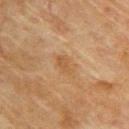The lesion was tiled from a total-body skin photograph and was not biopsied.
A female patient aged approximately 80.
Measured at roughly 2.5 mm in maximum diameter.
The lesion is on the upper back.
The tile uses cross-polarized illumination.
A lesion tile, about 15 mm wide, cut from a 3D total-body photograph.